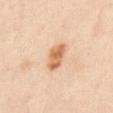Impression: Part of a total-body skin-imaging series; this lesion was reviewed on a skin check and was not flagged for biopsy. Background: A region of skin cropped from a whole-body photographic capture, roughly 15 mm wide. The lesion is on the abdomen. A male patient aged 63–67.